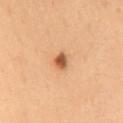  biopsy_status: not biopsied; imaged during a skin examination
  site: mid back
  patient:
    sex: female
    age_approx: 50
  lighting: cross-polarized
  image:
    source: total-body photography crop
    field_of_view_mm: 15
  lesion_size:
    long_diameter_mm_approx: 2.5